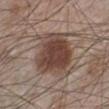Case summary:
- follow-up — total-body-photography surveillance lesion; no biopsy
- patient — male, aged approximately 60
- illumination — white-light illumination
- size — about 5.5 mm
- TBP lesion metrics — an outline eccentricity of about 0.5 (0 = round, 1 = elongated) and two-axis asymmetry of about 0.2; a border-irregularity rating of about 2/10 and a color-variation rating of about 4.5/10; a classifier nevus-likeness of about 100/100 and a detector confidence of about 100 out of 100 that the crop contains a lesion
- site — the left lower leg
- image source — ~15 mm tile from a whole-body skin photo